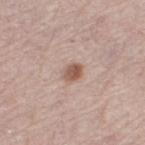| feature | finding |
|---|---|
| workup | catalogued during a skin exam; not biopsied |
| lesion diameter | ≈3 mm |
| lighting | white-light illumination |
| site | the right thigh |
| imaging modality | total-body-photography crop, ~15 mm field of view |
| subject | female, about 65 years old |